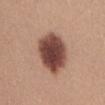notes = imaged on a skin check; not biopsied